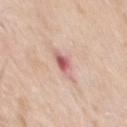Notes:
• site: the chest
• diameter: about 3.5 mm
• imaging modality: ~15 mm crop, total-body skin-cancer survey
• subject: male, in their 80s